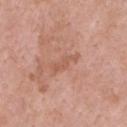Recorded during total-body skin imaging; not selected for excision or biopsy.
The lesion is on the chest.
A region of skin cropped from a whole-body photographic capture, roughly 15 mm wide.
The patient is a male aged 58 to 62.